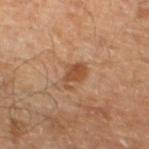anatomic site=the left lower leg
tile lighting=cross-polarized illumination
image=~15 mm crop, total-body skin-cancer survey
lesion size=≈3 mm
subject=male, aged 53 to 57